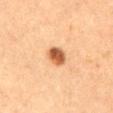<lesion>
<biopsy_status>not biopsied; imaged during a skin examination</biopsy_status>
<patient>
  <sex>female</sex>
  <age_approx>60</age_approx>
</patient>
<lesion_size>
  <long_diameter_mm_approx>2.5</long_diameter_mm_approx>
</lesion_size>
<site>abdomen</site>
<lighting>cross-polarized</lighting>
<image>
  <source>total-body photography crop</source>
  <field_of_view_mm>15</field_of_view_mm>
</image>
</lesion>